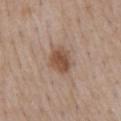No biopsy was performed on this lesion — it was imaged during a full skin examination and was not determined to be concerning. Captured under white-light illumination. The patient is a male aged approximately 75. The lesion is located on the mid back. This image is a 15 mm lesion crop taken from a total-body photograph.